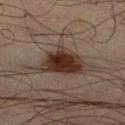{"lesion_size": {"long_diameter_mm_approx": 5.0}, "patient": {"sex": "male", "age_approx": 50}, "image": {"source": "total-body photography crop", "field_of_view_mm": 15}, "automated_metrics": {"nevus_likeness_0_100": 100, "lesion_detection_confidence_0_100": 100}, "site": "left leg"}